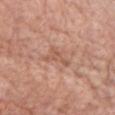Assessment: No biopsy was performed on this lesion — it was imaged during a full skin examination and was not determined to be concerning. Acquisition and patient details: The subject is a female aged 68–72. A 15 mm close-up tile from a total-body photography series done for melanoma screening. Located on the chest. The tile uses white-light illumination. The recorded lesion diameter is about 3.5 mm.